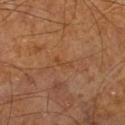Part of a total-body skin-imaging series; this lesion was reviewed on a skin check and was not flagged for biopsy. A male patient aged 58 to 62. Longest diameter approximately 2.5 mm. A region of skin cropped from a whole-body photographic capture, roughly 15 mm wide. Imaged with cross-polarized lighting. Located on the right lower leg. An algorithmic analysis of the crop reported a mean CIELAB color near L≈42 a*≈21 b*≈34 and a normalized lesion–skin contrast near 5. The software also gave a border-irregularity index near 5.5/10, internal color variation of about 0 on a 0–10 scale, and a peripheral color-asymmetry measure near 0. The analysis additionally found a classifier nevus-likeness of about 0/100 and a detector confidence of about 95 out of 100 that the crop contains a lesion.